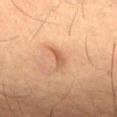lesion size: about 2.5 mm | site: the front of the torso | subject: male, about 55 years old | tile lighting: cross-polarized illumination | image-analysis metrics: a footprint of about 3 mm², a shape eccentricity near 0.9, and a symmetry-axis asymmetry near 0.45; border irregularity of about 4 on a 0–10 scale, a within-lesion color-variation index near 0.5/10, and peripheral color asymmetry of about 0; a lesion-detection confidence of about 95/100 | acquisition: 15 mm crop, total-body photography.